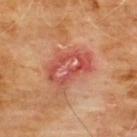The lesion was tiled from a total-body skin photograph and was not biopsied.
About 5.5 mm across.
From the chest.
A male subject aged 58 to 62.
A 15 mm close-up extracted from a 3D total-body photography capture.
Imaged with cross-polarized lighting.